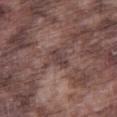Notes:
- notes — no biopsy performed (imaged during a skin exam)
- imaging modality — 15 mm crop, total-body photography
- subject — male, aged around 75
- lighting — white-light
- diameter — about 3 mm
- body site — the leg